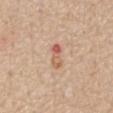Imaged during a routine full-body skin examination; the lesion was not biopsied and no histopathology is available.
Measured at roughly 3.5 mm in maximum diameter.
The tile uses white-light illumination.
The lesion is on the chest.
This image is a 15 mm lesion crop taken from a total-body photograph.
A male patient, aged around 65.
The lesion-visualizer software estimated an automated nevus-likeness rating near 0 out of 100 and a detector confidence of about 100 out of 100 that the crop contains a lesion.